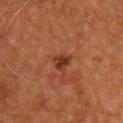An algorithmic analysis of the crop reported a footprint of about 4 mm² and a shape-asymmetry score of about 0.3 (0 = symmetric). The software also gave a mean CIELAB color near L≈34 a*≈25 b*≈32, about 9 CIELAB-L* units darker than the surrounding skin, and a lesion-to-skin contrast of about 8 (normalized; higher = more distinct). A male subject, about 55 years old. A 15 mm close-up extracted from a 3D total-body photography capture. Captured under cross-polarized illumination. The recorded lesion diameter is about 2.5 mm. On the upper back.